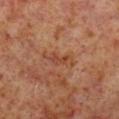Impression:
No biopsy was performed on this lesion — it was imaged during a full skin examination and was not determined to be concerning.
Background:
The tile uses cross-polarized illumination. Measured at roughly 4.5 mm in maximum diameter. From the right lower leg. A male patient, aged around 60. A close-up tile cropped from a whole-body skin photograph, about 15 mm across.